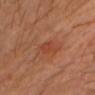Clinical impression:
Recorded during total-body skin imaging; not selected for excision or biopsy.
Image and clinical context:
About 2.5 mm across. A male subject in their 30s. A 15 mm crop from a total-body photograph taken for skin-cancer surveillance. The total-body-photography lesion software estimated a shape eccentricity near 0.7 and a shape-asymmetry score of about 0.25 (0 = symmetric). The software also gave roughly 5 lightness units darker than nearby skin and a normalized lesion–skin contrast near 4.5. The software also gave a border-irregularity index near 2.5/10. The lesion is on the chest.